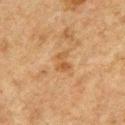Q: How large is the lesion?
A: ~2.5 mm (longest diameter)
Q: What kind of image is this?
A: total-body-photography crop, ~15 mm field of view
Q: Who is the patient?
A: male, in their mid-70s
Q: What lighting was used for the tile?
A: cross-polarized
Q: Where on the body is the lesion?
A: the front of the torso